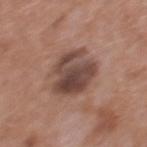This lesion was catalogued during total-body skin photography and was not selected for biopsy. An algorithmic analysis of the crop reported a lesion area of about 18 mm², an outline eccentricity of about 0.45 (0 = round, 1 = elongated), and a symmetry-axis asymmetry near 0.2. A lesion tile, about 15 mm wide, cut from a 3D total-body photograph. A male patient aged 68–72. From the mid back. Captured under white-light illumination.